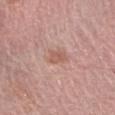<record>
<biopsy_status>not biopsied; imaged during a skin examination</biopsy_status>
<lesion_size>
  <long_diameter_mm_approx>2.5</long_diameter_mm_approx>
</lesion_size>
<site>left forearm</site>
<patient>
  <sex>female</sex>
  <age_approx>65</age_approx>
</patient>
<image>
  <source>total-body photography crop</source>
  <field_of_view_mm>15</field_of_view_mm>
</image>
<lighting>white-light</lighting>
</record>